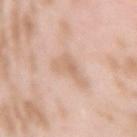A roughly 15 mm field-of-view crop from a total-body skin photograph.
The patient is a female aged 23–27.
Imaged with white-light lighting.
The lesion-visualizer software estimated a symmetry-axis asymmetry near 0.55. And it measured a border-irregularity index near 6.5/10.
Measured at roughly 4.5 mm in maximum diameter.
The lesion is located on the right upper arm.